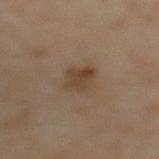Assessment:
The lesion was photographed on a routine skin check and not biopsied; there is no pathology result.
Acquisition and patient details:
Cropped from a total-body skin-imaging series; the visible field is about 15 mm. Imaged with cross-polarized lighting. The lesion is located on the mid back. The patient is a male in their mid- to late 60s. The recorded lesion diameter is about 3.5 mm.